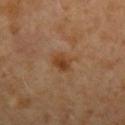Clinical impression:
This lesion was catalogued during total-body skin photography and was not selected for biopsy.
Clinical summary:
Located on the right forearm. A 15 mm close-up extracted from a 3D total-body photography capture. A female subject about 50 years old.